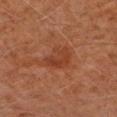Clinical impression: This lesion was catalogued during total-body skin photography and was not selected for biopsy. Clinical summary: A roughly 15 mm field-of-view crop from a total-body skin photograph. Captured under cross-polarized illumination. The lesion's longest dimension is about 4 mm. Located on the right upper arm. A male patient approximately 70 years of age.